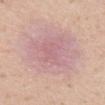Recorded during total-body skin imaging; not selected for excision or biopsy. From the upper back. A female patient, approximately 40 years of age. Measured at roughly 9 mm in maximum diameter. Imaged with white-light lighting. A 15 mm close-up extracted from a 3D total-body photography capture.